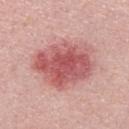| field | value |
|---|---|
| biopsy status | imaged on a skin check; not biopsied |
| acquisition | ~15 mm crop, total-body skin-cancer survey |
| subject | male, aged 28–32 |